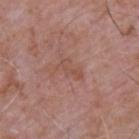Automated image analysis of the tile measured a mean CIELAB color near L≈51 a*≈22 b*≈27, a lesion–skin lightness drop of about 6, and a normalized border contrast of about 5.
A male patient, aged 63–67.
The lesion is located on the upper back.
Captured under white-light illumination.
About 3.5 mm across.
A 15 mm close-up tile from a total-body photography series done for melanoma screening.